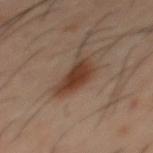workup = catalogued during a skin exam; not biopsied | site = the mid back | image = ~15 mm tile from a whole-body skin photo | subject = male, in their 40s | lighting = cross-polarized.